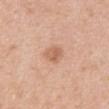<case>
  <automated_metrics>
    <area_mm2_approx>4.5</area_mm2_approx>
    <eccentricity>0.6</eccentricity>
    <shape_asymmetry>0.2</shape_asymmetry>
    <cielab_L>62</cielab_L>
    <cielab_a>22</cielab_a>
    <cielab_b>33</cielab_b>
    <vs_skin_contrast_norm>6.0</vs_skin_contrast_norm>
    <color_variation_0_10>2.5</color_variation_0_10>
    <peripheral_color_asymmetry>1.0</peripheral_color_asymmetry>
  </automated_metrics>
  <site>abdomen</site>
  <image>
    <source>total-body photography crop</source>
    <field_of_view_mm>15</field_of_view_mm>
  </image>
  <lighting>white-light</lighting>
  <lesion_size>
    <long_diameter_mm_approx>2.5</long_diameter_mm_approx>
  </lesion_size>
  <patient>
    <sex>female</sex>
    <age_approx>40</age_approx>
  </patient>
</case>